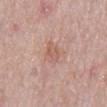The lesion was tiled from a total-body skin photograph and was not biopsied.